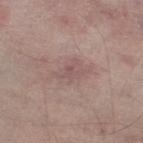subject: male, roughly 65 years of age; acquisition: 15 mm crop, total-body photography; location: the leg.